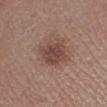Case summary:
- workup: imaged on a skin check; not biopsied
- lesion size: ~3.5 mm (longest diameter)
- imaging modality: ~15 mm crop, total-body skin-cancer survey
- site: the leg
- subject: male, roughly 50 years of age